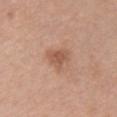{
  "biopsy_status": "not biopsied; imaged during a skin examination",
  "site": "chest",
  "image": {
    "source": "total-body photography crop",
    "field_of_view_mm": 15
  },
  "lesion_size": {
    "long_diameter_mm_approx": 3.0
  },
  "patient": {
    "sex": "female",
    "age_approx": 55
  },
  "automated_metrics": {
    "cielab_L": 55,
    "cielab_a": 22,
    "cielab_b": 30,
    "vs_skin_darker_L": 9.0,
    "vs_skin_contrast_norm": 6.5,
    "color_variation_0_10": 2.0,
    "peripheral_color_asymmetry": 0.5
  },
  "lighting": "white-light"
}